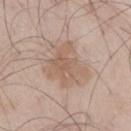{"lighting": "white-light", "image": {"source": "total-body photography crop", "field_of_view_mm": 15}, "site": "right thigh", "patient": {"sex": "male", "age_approx": 70}, "lesion_size": {"long_diameter_mm_approx": 5.5}}